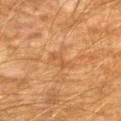Q: Was a biopsy performed?
A: catalogued during a skin exam; not biopsied
Q: What kind of image is this?
A: ~15 mm tile from a whole-body skin photo
Q: Where on the body is the lesion?
A: the left upper arm
Q: Patient demographics?
A: male, approximately 60 years of age